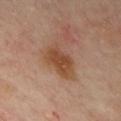Clinical impression: No biopsy was performed on this lesion — it was imaged during a full skin examination and was not determined to be concerning. Image and clinical context: The lesion is on the chest. A region of skin cropped from a whole-body photographic capture, roughly 15 mm wide. Imaged with cross-polarized lighting. An algorithmic analysis of the crop reported an outline eccentricity of about 0.8 (0 = round, 1 = elongated) and a symmetry-axis asymmetry near 0.25. A male subject, roughly 45 years of age.